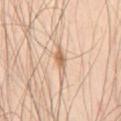Background:
The lesion is located on the lower back. A male patient in their mid- to late 40s. A lesion tile, about 15 mm wide, cut from a 3D total-body photograph. The lesion's longest dimension is about 3 mm.